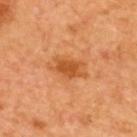Case summary:
• biopsy status · imaged on a skin check; not biopsied
• image · ~15 mm tile from a whole-body skin photo
• lesion diameter · ~4.5 mm (longest diameter)
• patient · male, approximately 65 years of age
• tile lighting · cross-polarized
• location · the upper back